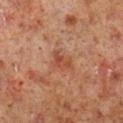Clinical impression: The lesion was tiled from a total-body skin photograph and was not biopsied. Image and clinical context: A 15 mm close-up tile from a total-body photography series done for melanoma screening. This is a cross-polarized tile. About 3 mm across. A male subject aged 58 to 62. Located on the right lower leg.